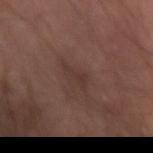The lesion was tiled from a total-body skin photograph and was not biopsied. Cropped from a total-body skin-imaging series; the visible field is about 15 mm. A male subject, in their mid-60s. From the left forearm.